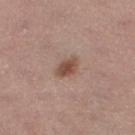No biopsy was performed on this lesion — it was imaged during a full skin examination and was not determined to be concerning.
Cropped from a total-body skin-imaging series; the visible field is about 15 mm.
Approximately 3 mm at its widest.
Captured under white-light illumination.
From the left lower leg.
A female subject aged around 55.